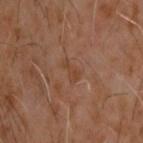<record>
  <biopsy_status>not biopsied; imaged during a skin examination</biopsy_status>
  <patient>
    <sex>male</sex>
    <age_approx>60</age_approx>
  </patient>
  <lighting>cross-polarized</lighting>
  <site>upper back</site>
  <image>
    <source>total-body photography crop</source>
    <field_of_view_mm>15</field_of_view_mm>
  </image>
</record>